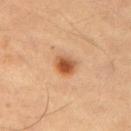Notes:
– subject — male, aged approximately 55
– acquisition — 15 mm crop, total-body photography
– tile lighting — cross-polarized illumination
– diameter — ~3 mm (longest diameter)
– site — the right upper arm
– automated lesion analysis — a nevus-likeness score of about 100/100 and a detector confidence of about 100 out of 100 that the crop contains a lesion